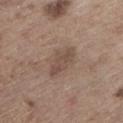workup: total-body-photography surveillance lesion; no biopsy
tile lighting: white-light
acquisition: ~15 mm crop, total-body skin-cancer survey
TBP lesion metrics: a shape eccentricity near 0.85; a mean CIELAB color near L≈48 a*≈16 b*≈24 and about 8 CIELAB-L* units darker than the surrounding skin; a border-irregularity rating of about 3/10 and a within-lesion color-variation index near 1.5/10; a nevus-likeness score of about 5/100 and a detector confidence of about 100 out of 100 that the crop contains a lesion
anatomic site: the left lower leg
lesion diameter: about 4 mm
subject: male, about 70 years old